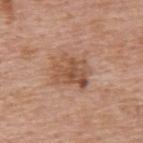  biopsy_status: not biopsied; imaged during a skin examination
  patient:
    sex: male
    age_approx: 75
  lesion_size:
    long_diameter_mm_approx: 4.5
  image:
    source: total-body photography crop
    field_of_view_mm: 15
  lighting: white-light
  automated_metrics:
    area_mm2_approx: 12.0
    eccentricity: 0.65
    vs_skin_contrast_norm: 7.0
    border_irregularity_0_10: 3.0
    lesion_detection_confidence_0_100: 100
  site: upper back